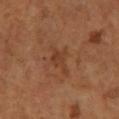The lesion was photographed on a routine skin check and not biopsied; there is no pathology result. A female subject roughly 65 years of age. The lesion is on the left lower leg. Automated tile analysis of the lesion measured an area of roughly 5.5 mm², an eccentricity of roughly 0.85, and a symmetry-axis asymmetry near 0.35. It also reported a classifier nevus-likeness of about 0/100 and a lesion-detection confidence of about 100/100. A 15 mm close-up extracted from a 3D total-body photography capture.